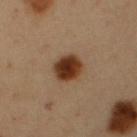follow-up: catalogued during a skin exam; not biopsied
diameter: ~3.5 mm (longest diameter)
automated metrics: an average lesion color of about L≈35 a*≈20 b*≈30 (CIELAB), a lesion–skin lightness drop of about 16, and a normalized border contrast of about 13.5; a border-irregularity index near 1/10, a within-lesion color-variation index near 5/10, and a peripheral color-asymmetry measure near 1.5
image source: total-body-photography crop, ~15 mm field of view
location: the abdomen
lighting: cross-polarized
patient: male, roughly 50 years of age